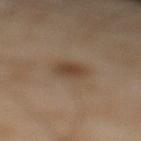| feature | finding |
|---|---|
| follow-up | catalogued during a skin exam; not biopsied |
| TBP lesion metrics | an average lesion color of about L≈42 a*≈15 b*≈28 (CIELAB) and a normalized border contrast of about 7.5; a border-irregularity rating of about 2/10 and a color-variation rating of about 2.5/10; an automated nevus-likeness rating near 80 out of 100 |
| lighting | cross-polarized illumination |
| image | 15 mm crop, total-body photography |
| lesion size | ≈2.5 mm |
| body site | the left lower leg |
| patient | male, about 55 years old |